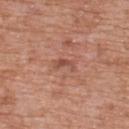Case summary:
* notes — no biopsy performed (imaged during a skin exam)
* patient — male, aged around 75
* image source — 15 mm crop, total-body photography
* lighting — white-light
* anatomic site — the back
* lesion size — ~3 mm (longest diameter)